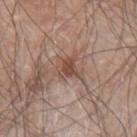<record>
<biopsy_status>not biopsied; imaged during a skin examination</biopsy_status>
<patient>
  <sex>male</sex>
  <age_approx>80</age_approx>
</patient>
<automated_metrics>
  <area_mm2_approx>6.0</area_mm2_approx>
  <eccentricity>0.65</eccentricity>
  <shape_asymmetry>0.4</shape_asymmetry>
  <border_irregularity_0_10>4.5</border_irregularity_0_10>
  <color_variation_0_10>2.5</color_variation_0_10>
</automated_metrics>
<lighting>white-light</lighting>
<image>
  <source>total-body photography crop</source>
  <field_of_view_mm>15</field_of_view_mm>
</image>
<site>left arm</site>
</record>